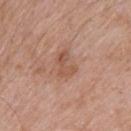The lesion was photographed on a routine skin check and not biopsied; there is no pathology result. The lesion is on the upper back. A 15 mm close-up tile from a total-body photography series done for melanoma screening. A male patient aged around 55. The lesion-visualizer software estimated an area of roughly 5 mm² and a symmetry-axis asymmetry near 0.3. It also reported a mean CIELAB color near L≈54 a*≈22 b*≈30, roughly 8 lightness units darker than nearby skin, and a normalized border contrast of about 6. The analysis additionally found radial color variation of about 1.5.